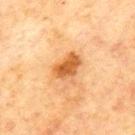notes: catalogued during a skin exam; not biopsied | site: the back | imaging modality: ~15 mm crop, total-body skin-cancer survey | lesion size: ≈3.5 mm | image-analysis metrics: an area of roughly 7 mm², a shape eccentricity near 0.8, and a shape-asymmetry score of about 0.2 (0 = symmetric); an average lesion color of about L≈50 a*≈23 b*≈40 (CIELAB) and a normalized border contrast of about 9.5; a border-irregularity rating of about 2/10, internal color variation of about 2.5 on a 0–10 scale, and peripheral color asymmetry of about 1 | patient: male, aged around 70 | lighting: cross-polarized.